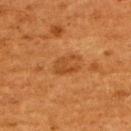Part of a total-body skin-imaging series; this lesion was reviewed on a skin check and was not flagged for biopsy.
Approximately 3 mm at its widest.
Located on the back.
This image is a 15 mm lesion crop taken from a total-body photograph.
The total-body-photography lesion software estimated a footprint of about 5 mm², an eccentricity of roughly 0.65, and a symmetry-axis asymmetry near 0.4. And it measured a border-irregularity rating of about 5/10, a color-variation rating of about 1.5/10, and peripheral color asymmetry of about 0.5.
A female subject, aged 48–52.